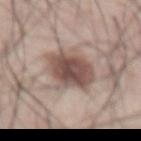<case>
<biopsy_status>not biopsied; imaged during a skin examination</biopsy_status>
<patient>
  <sex>male</sex>
  <age_approx>65</age_approx>
</patient>
<lesion_size>
  <long_diameter_mm_approx>5.5</long_diameter_mm_approx>
</lesion_size>
<site>front of the torso</site>
<image>
  <source>total-body photography crop</source>
  <field_of_view_mm>15</field_of_view_mm>
</image>
</case>